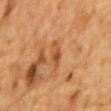biopsy_status: not biopsied; imaged during a skin examination
automated_metrics:
  color_variation_0_10: 3.5
  peripheral_color_asymmetry: 1.5
lesion_size:
  long_diameter_mm_approx: 3.5
patient:
  sex: male
  age_approx: 85+
image:
  source: total-body photography crop
  field_of_view_mm: 15
site: chest